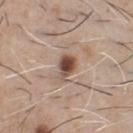Recorded during total-body skin imaging; not selected for excision or biopsy.
A male patient approximately 60 years of age.
The lesion-visualizer software estimated an area of roughly 5.5 mm² and a shape eccentricity near 0.8.
Located on the chest.
The tile uses white-light illumination.
This image is a 15 mm lesion crop taken from a total-body photograph.
The recorded lesion diameter is about 3.5 mm.